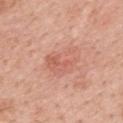Recorded during total-body skin imaging; not selected for excision or biopsy. A female subject, aged around 50. The lesion's longest dimension is about 4 mm. From the upper back. This image is a 15 mm lesion crop taken from a total-body photograph. This is a white-light tile.